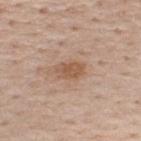Assessment:
The lesion was photographed on a routine skin check and not biopsied; there is no pathology result.
Background:
A male patient, aged approximately 60. The tile uses white-light illumination. A 15 mm close-up extracted from a 3D total-body photography capture. Located on the upper back.